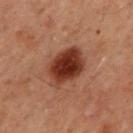{
  "biopsy_status": "not biopsied; imaged during a skin examination",
  "image": {
    "source": "total-body photography crop",
    "field_of_view_mm": 15
  },
  "patient": {
    "sex": "male",
    "age_approx": 60
  },
  "site": "back"
}